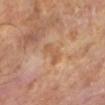| key | value |
|---|---|
| lesion size | ~3.5 mm (longest diameter) |
| subject | male, in their mid- to late 60s |
| lighting | cross-polarized |
| automated lesion analysis | an average lesion color of about L≈55 a*≈20 b*≈34 (CIELAB), roughly 7 lightness units darker than nearby skin, and a normalized lesion–skin contrast near 5.5; a classifier nevus-likeness of about 0/100 |
| imaging modality | ~15 mm crop, total-body skin-cancer survey |